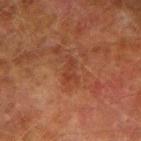Q: Patient demographics?
A: male, in their mid-70s
Q: Lesion size?
A: ~3 mm (longest diameter)
Q: Where on the body is the lesion?
A: the right upper arm
Q: How was this image acquired?
A: ~15 mm crop, total-body skin-cancer survey
Q: How was the tile lit?
A: cross-polarized